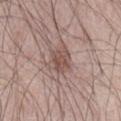Assessment:
No biopsy was performed on this lesion — it was imaged during a full skin examination and was not determined to be concerning.
Clinical summary:
From the abdomen. The lesion-visualizer software estimated a lesion area of about 7.5 mm², a shape eccentricity near 0.6, and a shape-asymmetry score of about 0.25 (0 = symmetric). The software also gave radial color variation of about 1. The lesion's longest dimension is about 3.5 mm. Imaged with white-light lighting. A region of skin cropped from a whole-body photographic capture, roughly 15 mm wide. The subject is a male approximately 65 years of age.